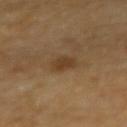biopsy status: no biopsy performed (imaged during a skin exam)
anatomic site: the left forearm
tile lighting: cross-polarized illumination
patient: female, in their 70s
image: total-body-photography crop, ~15 mm field of view
size: about 3 mm